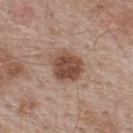biopsy status — imaged on a skin check; not biopsied
lighting — white-light
subject — male, aged 53–57
lesion diameter — ≈4 mm
image source — ~15 mm tile from a whole-body skin photo
location — the upper back